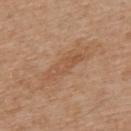Notes:
– biopsy status · catalogued during a skin exam; not biopsied
– subject · male, in their 70s
– image source · 15 mm crop, total-body photography
– TBP lesion metrics · a footprint of about 8 mm², an outline eccentricity of about 0.95 (0 = round, 1 = elongated), and two-axis asymmetry of about 0.3; an average lesion color of about L≈53 a*≈20 b*≈34 (CIELAB) and about 7 CIELAB-L* units darker than the surrounding skin
– lesion diameter · about 5.5 mm
– location · the upper back
– illumination · white-light illumination